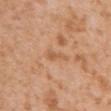<lesion>
<biopsy_status>not biopsied; imaged during a skin examination</biopsy_status>
<patient>
  <sex>female</sex>
  <age_approx>30</age_approx>
</patient>
<image>
  <source>total-body photography crop</source>
  <field_of_view_mm>15</field_of_view_mm>
</image>
<lesion_size>
  <long_diameter_mm_approx>3.5</long_diameter_mm_approx>
</lesion_size>
<lighting>white-light</lighting>
<site>left upper arm</site>
</lesion>